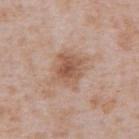Clinical impression:
Imaged during a routine full-body skin examination; the lesion was not biopsied and no histopathology is available.
Context:
A 15 mm close-up tile from a total-body photography series done for melanoma screening. A male subject about 65 years old. From the abdomen.